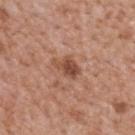Assessment:
This lesion was catalogued during total-body skin photography and was not selected for biopsy.
Clinical summary:
The tile uses white-light illumination. Approximately 3.5 mm at its widest. A male patient about 65 years old. On the mid back. Cropped from a whole-body photographic skin survey; the tile spans about 15 mm. Automated image analysis of the tile measured an average lesion color of about L≈48 a*≈23 b*≈29 (CIELAB), a lesion–skin lightness drop of about 12, and a normalized lesion–skin contrast near 8.5.